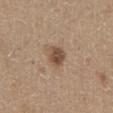The lesion was photographed on a routine skin check and not biopsied; there is no pathology result.
Located on the abdomen.
A roughly 15 mm field-of-view crop from a total-body skin photograph.
The tile uses white-light illumination.
A male patient, about 65 years old.
Measured at roughly 2.5 mm in maximum diameter.